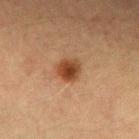Notes:
* notes: imaged on a skin check; not biopsied
* subject: female, aged around 55
* acquisition: total-body-photography crop, ~15 mm field of view
* location: the left forearm